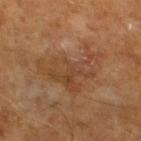– follow-up — total-body-photography surveillance lesion; no biopsy
– acquisition — ~15 mm crop, total-body skin-cancer survey
– tile lighting — cross-polarized illumination
– patient — male, about 65 years old
– image-analysis metrics — a lesion color around L≈43 a*≈20 b*≈32 in CIELAB and roughly 7 lightness units darker than nearby skin; a border-irregularity index near 8/10, internal color variation of about 5 on a 0–10 scale, and radial color variation of about 1.5; an automated nevus-likeness rating near 0 out of 100 and lesion-presence confidence of about 100/100
– size — about 7 mm